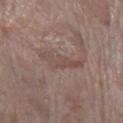  biopsy_status: not biopsied; imaged during a skin examination
  image:
    source: total-body photography crop
    field_of_view_mm: 15
  site: left lower leg
  patient:
    sex: female
    age_approx: 70